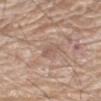image source = ~15 mm crop, total-body skin-cancer survey; subject = male, about 80 years old; illumination = white-light; size = about 3.5 mm; location = the arm.